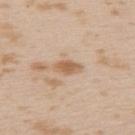Impression: No biopsy was performed on this lesion — it was imaged during a full skin examination and was not determined to be concerning. Clinical summary: The subject is a female in their mid- to late 20s. Imaged with white-light lighting. This image is a 15 mm lesion crop taken from a total-body photograph. About 3.5 mm across. The lesion is located on the upper back.